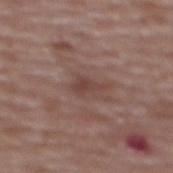{
  "biopsy_status": "not biopsied; imaged during a skin examination",
  "lesion_size": {
    "long_diameter_mm_approx": 3.5
  },
  "image": {
    "source": "total-body photography crop",
    "field_of_view_mm": 15
  },
  "site": "upper back",
  "lighting": "white-light",
  "patient": {
    "sex": "female",
    "age_approx": 65
  }
}